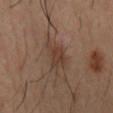Findings:
– notes · catalogued during a skin exam; not biopsied
– subject · male, in their 40s
– location · the mid back
– size · ≈4.5 mm
– lighting · cross-polarized
– image-analysis metrics · a lesion area of about 8 mm²; a border-irregularity index near 4.5/10, internal color variation of about 4.5 on a 0–10 scale, and peripheral color asymmetry of about 2
– image · total-body-photography crop, ~15 mm field of view